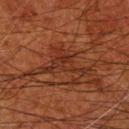Imaged during a routine full-body skin examination; the lesion was not biopsied and no histopathology is available.
A male subject, aged approximately 80.
This is a cross-polarized tile.
Longest diameter approximately 6 mm.
This image is a 15 mm lesion crop taken from a total-body photograph.
The lesion is on the left forearm.